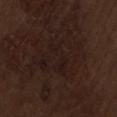Q: Was this lesion biopsied?
A: total-body-photography surveillance lesion; no biopsy
Q: What kind of image is this?
A: ~15 mm tile from a whole-body skin photo
Q: What are the patient's age and sex?
A: male, approximately 70 years of age
Q: What is the anatomic site?
A: the back